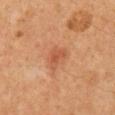Recorded during total-body skin imaging; not selected for excision or biopsy. On the mid back. Automated image analysis of the tile measured a mean CIELAB color near L≈48 a*≈25 b*≈33 and a normalized lesion–skin contrast near 6. It also reported a border-irregularity index near 4/10, internal color variation of about 2 on a 0–10 scale, and peripheral color asymmetry of about 0.5. The subject is a male aged 58 to 62. A lesion tile, about 15 mm wide, cut from a 3D total-body photograph.